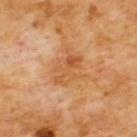Captured during whole-body skin photography for melanoma surveillance; the lesion was not biopsied. On the chest. Approximately 4.5 mm at its widest. A male patient, aged 58 to 62. A 15 mm crop from a total-body photograph taken for skin-cancer surveillance.